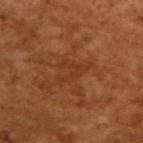biopsy status — imaged on a skin check; not biopsied
tile lighting — cross-polarized illumination
lesion diameter — about 4 mm
subject — male, about 65 years old
TBP lesion metrics — an eccentricity of roughly 0.75 and a symmetry-axis asymmetry near 0.7; a nevus-likeness score of about 0/100 and lesion-presence confidence of about 95/100
acquisition — ~15 mm crop, total-body skin-cancer survey